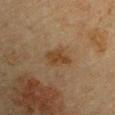The lesion was tiled from a total-body skin photograph and was not biopsied.
A close-up tile cropped from a whole-body skin photograph, about 15 mm across.
Automated image analysis of the tile measured a mean CIELAB color near L≈33 a*≈14 b*≈28 and about 7 CIELAB-L* units darker than the surrounding skin. It also reported a border-irregularity index near 2.5/10 and a peripheral color-asymmetry measure near 1. The software also gave a nevus-likeness score of about 70/100 and a lesion-detection confidence of about 100/100.
The lesion's longest dimension is about 3 mm.
Located on the right upper arm.
A male subject roughly 65 years of age.
Imaged with cross-polarized lighting.